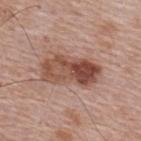workup: total-body-photography surveillance lesion; no biopsy
size: ≈6.5 mm
image source: total-body-photography crop, ~15 mm field of view
tile lighting: white-light
patient: male, in their mid- to late 60s
location: the upper back
automated metrics: a border-irregularity rating of about 2.5/10 and a color-variation rating of about 9/10; a nevus-likeness score of about 25/100 and a detector confidence of about 100 out of 100 that the crop contains a lesion